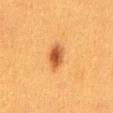The lesion was photographed on a routine skin check and not biopsied; there is no pathology result. This image is a 15 mm lesion crop taken from a total-body photograph. Measured at roughly 3.5 mm in maximum diameter. On the mid back. A female subject, approximately 40 years of age.